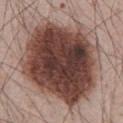- notes · imaged on a skin check; not biopsied
- imaging modality · total-body-photography crop, ~15 mm field of view
- body site · the abdomen
- subject · male, aged 63 to 67